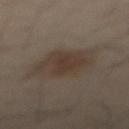workup: catalogued during a skin exam; not biopsied
image-analysis metrics: an average lesion color of about L≈37 a*≈11 b*≈22 (CIELAB); a border-irregularity rating of about 2/10 and peripheral color asymmetry of about 0.5
diameter: about 5 mm
site: the abdomen
tile lighting: cross-polarized
image: ~15 mm tile from a whole-body skin photo